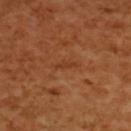workup: total-body-photography surveillance lesion; no biopsy
subject: female, aged 53–57
anatomic site: the back
acquisition: total-body-photography crop, ~15 mm field of view